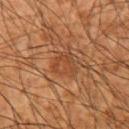Q: Was a biopsy performed?
A: no biopsy performed (imaged during a skin exam)
Q: What is the anatomic site?
A: the right upper arm
Q: Automated lesion metrics?
A: a mean CIELAB color near L≈35 a*≈20 b*≈29, a lesion–skin lightness drop of about 5, and a lesion-to-skin contrast of about 5 (normalized; higher = more distinct)
Q: Patient demographics?
A: male, in their mid- to late 60s
Q: What is the imaging modality?
A: ~15 mm tile from a whole-body skin photo